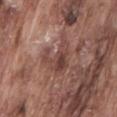The lesion was tiled from a total-body skin photograph and was not biopsied. The lesion is on the lower back. The tile uses white-light illumination. Measured at roughly 5 mm in maximum diameter. The patient is a male about 75 years old. The lesion-visualizer software estimated a shape eccentricity near 0.5 and a symmetry-axis asymmetry near 0.6. It also reported a border-irregularity rating of about 7/10. The software also gave an automated nevus-likeness rating near 0 out of 100 and lesion-presence confidence of about 100/100. A lesion tile, about 15 mm wide, cut from a 3D total-body photograph.